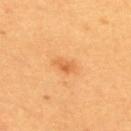body site: the upper back
size: about 3 mm
patient: female, aged 23–27
acquisition: total-body-photography crop, ~15 mm field of view
illumination: cross-polarized illumination
automated metrics: a lesion area of about 3.5 mm² and a shape eccentricity near 0.8; a mean CIELAB color near L≈63 a*≈27 b*≈46, about 9 CIELAB-L* units darker than the surrounding skin, and a lesion-to-skin contrast of about 5.5 (normalized; higher = more distinct)